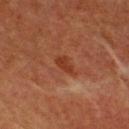The lesion was tiled from a total-body skin photograph and was not biopsied.
A region of skin cropped from a whole-body photographic capture, roughly 15 mm wide.
About 3 mm across.
This is a cross-polarized tile.
Located on the chest.
A male subject about 75 years old.